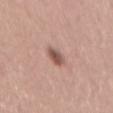The lesion was tiled from a total-body skin photograph and was not biopsied.
Measured at roughly 3 mm in maximum diameter.
A female patient aged 53–57.
Cropped from a whole-body photographic skin survey; the tile spans about 15 mm.
The lesion is on the mid back.
The tile uses white-light illumination.